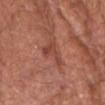The lesion was tiled from a total-body skin photograph and was not biopsied.
The lesion is on the head or neck.
A region of skin cropped from a whole-body photographic capture, roughly 15 mm wide.
The patient is a male aged around 80.
An algorithmic analysis of the crop reported a border-irregularity index near 6.5/10, a within-lesion color-variation index near 4/10, and peripheral color asymmetry of about 1.5. The software also gave a detector confidence of about 100 out of 100 that the crop contains a lesion.
This is a white-light tile.
Approximately 4 mm at its widest.